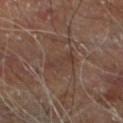Notes:
* TBP lesion metrics — an area of roughly 4.5 mm² and an outline eccentricity of about 0.9 (0 = round, 1 = elongated); a classifier nevus-likeness of about 0/100 and a detector confidence of about 85 out of 100 that the crop contains a lesion
* subject — male, aged approximately 70
* size — about 3.5 mm
* image — total-body-photography crop, ~15 mm field of view
* location — the left lower leg